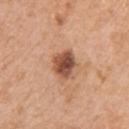  lighting: white-light
  patient:
    sex: female
    age_approx: 55
  lesion_size:
    long_diameter_mm_approx: 3.5
  site: right upper arm
  image:
    source: total-body photography crop
    field_of_view_mm: 15
  automated_metrics:
    cielab_L: 51
    cielab_a: 24
    cielab_b: 32
    vs_skin_darker_L: 16.0
    vs_skin_contrast_norm: 10.5
    border_irregularity_0_10: 2.5
    peripheral_color_asymmetry: 2.0